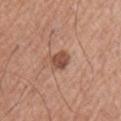Q: Is there a histopathology result?
A: no biopsy performed (imaged during a skin exam)
Q: Lesion location?
A: the left upper arm
Q: What kind of image is this?
A: ~15 mm tile from a whole-body skin photo
Q: How was the tile lit?
A: white-light illumination
Q: Who is the patient?
A: male, aged 63 to 67